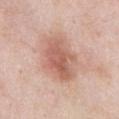Clinical impression:
Part of a total-body skin-imaging series; this lesion was reviewed on a skin check and was not flagged for biopsy.
Background:
Approximately 5.5 mm at its widest. Automated image analysis of the tile measured a footprint of about 17 mm², an eccentricity of roughly 0.75, and a symmetry-axis asymmetry near 0.2. And it measured a mean CIELAB color near L≈60 a*≈23 b*≈28, about 11 CIELAB-L* units darker than the surrounding skin, and a lesion-to-skin contrast of about 7 (normalized; higher = more distinct). This is a white-light tile. A male subject aged around 55. The lesion is on the front of the torso. A roughly 15 mm field-of-view crop from a total-body skin photograph.